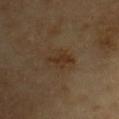Clinical impression:
The lesion was tiled from a total-body skin photograph and was not biopsied.
Acquisition and patient details:
The lesion is on the chest. The subject is a male aged 83 or older. A roughly 15 mm field-of-view crop from a total-body skin photograph.